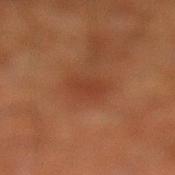{"biopsy_status": "not biopsied; imaged during a skin examination", "site": "left lower leg", "patient": {"sex": "male", "age_approx": 65}, "lighting": "cross-polarized", "image": {"source": "total-body photography crop", "field_of_view_mm": 15}, "lesion_size": {"long_diameter_mm_approx": 3.0}}